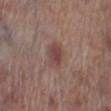Recorded during total-body skin imaging; not selected for excision or biopsy. Cropped from a whole-body photographic skin survey; the tile spans about 15 mm. Imaged with white-light lighting. The lesion is on the right lower leg. A male patient approximately 70 years of age.